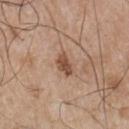Recorded during total-body skin imaging; not selected for excision or biopsy. Cropped from a total-body skin-imaging series; the visible field is about 15 mm. The lesion is on the chest. A male patient aged around 65.